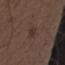anatomic site — the arm
image source — 15 mm crop, total-body photography
subject — male, roughly 50 years of age